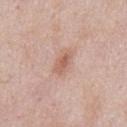notes = total-body-photography surveillance lesion; no biopsy
size = ≈3.5 mm
patient = male, in their mid-50s
automated metrics = a footprint of about 5 mm², a shape eccentricity near 0.85, and two-axis asymmetry of about 0.2; a mean CIELAB color near L≈60 a*≈22 b*≈28, a lesion–skin lightness drop of about 9, and a normalized border contrast of about 6.5
tile lighting = white-light
acquisition = ~15 mm tile from a whole-body skin photo
location = the abdomen